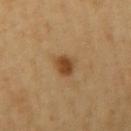Q: Is there a histopathology result?
A: catalogued during a skin exam; not biopsied